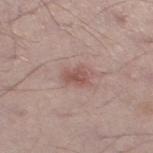• workup · no biopsy performed (imaged during a skin exam)
• lighting · white-light illumination
• subject · male, in their mid-50s
• TBP lesion metrics · a footprint of about 4.5 mm² and a shape-asymmetry score of about 0.35 (0 = symmetric); an average lesion color of about L≈52 a*≈21 b*≈23 (CIELAB), about 10 CIELAB-L* units darker than the surrounding skin, and a normalized lesion–skin contrast near 7; a border-irregularity rating of about 3/10 and peripheral color asymmetry of about 1; a classifier nevus-likeness of about 15/100 and a lesion-detection confidence of about 100/100
• site · the leg
• imaging modality · ~15 mm tile from a whole-body skin photo
• size · ≈2.5 mm